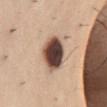The lesion was photographed on a routine skin check and not biopsied; there is no pathology result.
A 15 mm crop from a total-body photograph taken for skin-cancer surveillance.
The lesion is located on the abdomen.
A male subject, aged 33–37.
The tile uses white-light illumination.
Automated tile analysis of the lesion measured an area of roughly 14 mm² and a shape eccentricity near 0.55. The analysis additionally found a border-irregularity index near 1/10, a within-lesion color-variation index near 8/10, and a peripheral color-asymmetry measure near 2. The software also gave a nevus-likeness score of about 70/100 and a detector confidence of about 100 out of 100 that the crop contains a lesion.